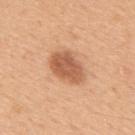Recorded during total-body skin imaging; not selected for excision or biopsy.